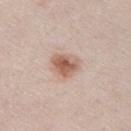– workup: catalogued during a skin exam; not biopsied
– acquisition: ~15 mm crop, total-body skin-cancer survey
– anatomic site: the right upper arm
– size: ~3.5 mm (longest diameter)
– automated lesion analysis: an automated nevus-likeness rating near 95 out of 100 and lesion-presence confidence of about 100/100
– patient: male, aged approximately 40